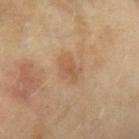<case>
  <biopsy_status>not biopsied; imaged during a skin examination</biopsy_status>
</case>